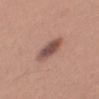notes: catalogued during a skin exam; not biopsied
size: about 4.5 mm
body site: the mid back
tile lighting: white-light illumination
imaging modality: ~15 mm tile from a whole-body skin photo
patient: male, about 30 years old